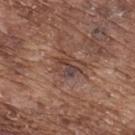biopsy status=no biopsy performed (imaged during a skin exam)